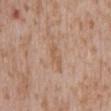Q: Was this lesion biopsied?
A: no biopsy performed (imaged during a skin exam)